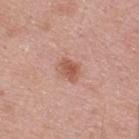  biopsy_status: not biopsied; imaged during a skin examination
  patient:
    sex: male
    age_approx: 45
  site: back
  lighting: white-light
  image:
    source: total-body photography crop
    field_of_view_mm: 15
  lesion_size:
    long_diameter_mm_approx: 3.0